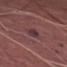Assessment: Recorded during total-body skin imaging; not selected for excision or biopsy. Clinical summary: From the left thigh. The patient is a male aged 73 to 77. Automated tile analysis of the lesion measured an area of roughly 4 mm², an eccentricity of roughly 0.9, and a shape-asymmetry score of about 0.25 (0 = symmetric). The analysis additionally found a border-irregularity index near 3/10, a color-variation rating of about 2/10, and radial color variation of about 0.5. It also reported a lesion-detection confidence of about 95/100. A close-up tile cropped from a whole-body skin photograph, about 15 mm across.